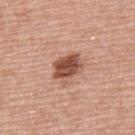workup — no biopsy performed (imaged during a skin exam)
anatomic site — the upper back
TBP lesion metrics — a border-irregularity rating of about 3/10 and a color-variation rating of about 3.5/10; a nevus-likeness score of about 45/100 and a lesion-detection confidence of about 100/100
size — ~4 mm (longest diameter)
lighting — white-light illumination
subject — male, about 70 years old
imaging modality — total-body-photography crop, ~15 mm field of view